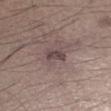Impression:
Imaged during a routine full-body skin examination; the lesion was not biopsied and no histopathology is available.
Clinical summary:
This is a white-light tile. A close-up tile cropped from a whole-body skin photograph, about 15 mm across. About 2.5 mm across. The subject is a male aged 33–37. From the leg.